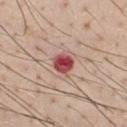Context:
The total-body-photography lesion software estimated an area of roughly 5.5 mm². And it measured an automated nevus-likeness rating near 0 out of 100 and a detector confidence of about 100 out of 100 that the crop contains a lesion. Imaged with white-light lighting. A lesion tile, about 15 mm wide, cut from a 3D total-body photograph. The lesion is on the front of the torso. The subject is a male aged 38–42.